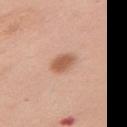  biopsy_status: not biopsied; imaged during a skin examination
  lesion_size:
    long_diameter_mm_approx: 3.0
  patient:
    sex: female
    age_approx: 50
  image:
    source: total-body photography crop
    field_of_view_mm: 15
  lighting: white-light
  site: left upper arm
  automated_metrics:
    area_mm2_approx: 6.0
    eccentricity: 0.65
    shape_asymmetry: 0.2